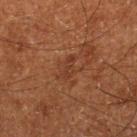workup: catalogued during a skin exam; not biopsied.